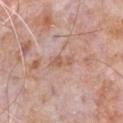follow-up: catalogued during a skin exam; not biopsied
tile lighting: white-light illumination
location: the chest
patient: male, aged approximately 80
image source: total-body-photography crop, ~15 mm field of view